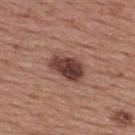Clinical impression:
The lesion was photographed on a routine skin check and not biopsied; there is no pathology result.
Image and clinical context:
A male patient roughly 55 years of age. Cropped from a whole-body photographic skin survey; the tile spans about 15 mm. Captured under white-light illumination. An algorithmic analysis of the crop reported a lesion color around L≈40 a*≈22 b*≈24 in CIELAB, a lesion–skin lightness drop of about 16, and a normalized border contrast of about 12. The software also gave a border-irregularity rating of about 2/10. The recorded lesion diameter is about 4 mm. On the upper back.